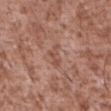• notes · catalogued during a skin exam; not biopsied
• subject · male, in their mid- to late 40s
• body site · the abdomen
• image source · total-body-photography crop, ~15 mm field of view